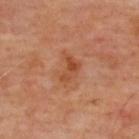No biopsy was performed on this lesion — it was imaged during a full skin examination and was not determined to be concerning.
Cropped from a total-body skin-imaging series; the visible field is about 15 mm.
Approximately 3.5 mm at its widest.
The lesion-visualizer software estimated an average lesion color of about L≈47 a*≈26 b*≈35 (CIELAB) and a lesion-to-skin contrast of about 7 (normalized; higher = more distinct). The analysis additionally found internal color variation of about 5 on a 0–10 scale.
The subject is a male in their mid-60s.
From the back.